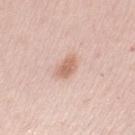follow-up: imaged on a skin check; not biopsied | TBP lesion metrics: a footprint of about 4.5 mm², an eccentricity of roughly 0.75, and two-axis asymmetry of about 0.2; a mean CIELAB color near L≈65 a*≈20 b*≈29, about 11 CIELAB-L* units darker than the surrounding skin, and a normalized border contrast of about 7; a border-irregularity index near 2/10 and peripheral color asymmetry of about 0.5; a nevus-likeness score of about 75/100 and a lesion-detection confidence of about 100/100 | body site: the left upper arm | patient: female, aged 28–32 | diameter: about 3 mm | acquisition: total-body-photography crop, ~15 mm field of view | tile lighting: white-light.